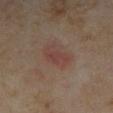No biopsy was performed on this lesion — it was imaged during a full skin examination and was not determined to be concerning.
The subject is a female approximately 35 years of age.
A 15 mm crop from a total-body photograph taken for skin-cancer surveillance.
The total-body-photography lesion software estimated a mean CIELAB color near L≈39 a*≈18 b*≈22, about 5 CIELAB-L* units darker than the surrounding skin, and a normalized border contrast of about 5. The software also gave a classifier nevus-likeness of about 80/100.
From the left forearm.
Measured at roughly 3.5 mm in maximum diameter.